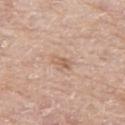Q: Was a biopsy performed?
A: total-body-photography surveillance lesion; no biopsy
Q: How was this image acquired?
A: ~15 mm crop, total-body skin-cancer survey
Q: How large is the lesion?
A: about 2.5 mm
Q: Lesion location?
A: the right thigh
Q: Illumination type?
A: white-light
Q: What are the patient's age and sex?
A: female, aged 73–77